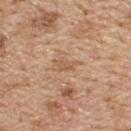Q: Is there a histopathology result?
A: no biopsy performed (imaged during a skin exam)
Q: Lesion location?
A: the upper back
Q: What lighting was used for the tile?
A: white-light illumination
Q: What is the imaging modality?
A: ~15 mm tile from a whole-body skin photo
Q: Lesion size?
A: ≈2.5 mm
Q: Who is the patient?
A: male, approximately 70 years of age